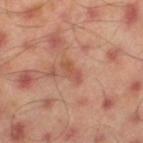| field | value |
|---|---|
| biopsy status | catalogued during a skin exam; not biopsied |
| site | the leg |
| lesion size | ≈3 mm |
| imaging modality | total-body-photography crop, ~15 mm field of view |
| patient | male, about 45 years old |
| illumination | cross-polarized |
| TBP lesion metrics | a lesion area of about 3.5 mm², an eccentricity of roughly 0.9, and a symmetry-axis asymmetry near 0.3; a border-irregularity rating of about 3/10 and a within-lesion color-variation index near 1/10; a nevus-likeness score of about 0/100 and a lesion-detection confidence of about 100/100 |